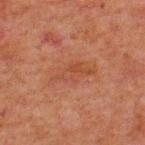Recorded during total-body skin imaging; not selected for excision or biopsy.
Automated image analysis of the tile measured an outline eccentricity of about 0.9 (0 = round, 1 = elongated) and two-axis asymmetry of about 0.35. And it measured a lesion color around L≈36 a*≈21 b*≈27 in CIELAB, roughly 5 lightness units darker than nearby skin, and a lesion-to-skin contrast of about 4.5 (normalized; higher = more distinct). And it measured a color-variation rating of about 3.5/10 and peripheral color asymmetry of about 1.5.
Measured at roughly 5.5 mm in maximum diameter.
Imaged with cross-polarized lighting.
The lesion is on the upper back.
The subject is a male approximately 60 years of age.
This image is a 15 mm lesion crop taken from a total-body photograph.